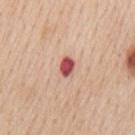Q: Was a biopsy performed?
A: imaged on a skin check; not biopsied
Q: How was this image acquired?
A: ~15 mm tile from a whole-body skin photo
Q: What are the patient's age and sex?
A: male, aged 58 to 62
Q: How was the tile lit?
A: white-light
Q: What is the anatomic site?
A: the mid back
Q: Lesion size?
A: ~2.5 mm (longest diameter)